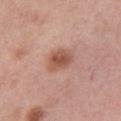workup: imaged on a skin check; not biopsied
automated metrics: a color-variation rating of about 3.5/10
location: the right thigh
diameter: ≈3.5 mm
imaging modality: ~15 mm crop, total-body skin-cancer survey
subject: female, about 50 years old